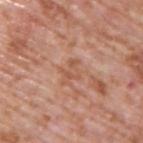Recorded during total-body skin imaging; not selected for excision or biopsy.
From the upper back.
This image is a 15 mm lesion crop taken from a total-body photograph.
The subject is a male in their 70s.